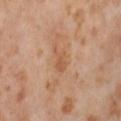| field | value |
|---|---|
| follow-up | total-body-photography surveillance lesion; no biopsy |
| diameter | ~3 mm (longest diameter) |
| tile lighting | cross-polarized |
| patient | female, aged 53 to 57 |
| site | the front of the torso |
| image-analysis metrics | a lesion area of about 4 mm², an outline eccentricity of about 0.75 (0 = round, 1 = elongated), and a symmetry-axis asymmetry near 0.45; an average lesion color of about L≈57 a*≈22 b*≈35 (CIELAB) and a lesion-to-skin contrast of about 5.5 (normalized; higher = more distinct); internal color variation of about 2 on a 0–10 scale and a peripheral color-asymmetry measure near 0.5; a nevus-likeness score of about 0/100 and lesion-presence confidence of about 100/100 |
| image | 15 mm crop, total-body photography |